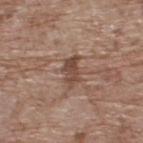Imaged during a routine full-body skin examination; the lesion was not biopsied and no histopathology is available. A male subject about 70 years old. The lesion is located on the upper back. A lesion tile, about 15 mm wide, cut from a 3D total-body photograph.